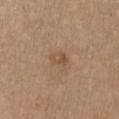Context:
The recorded lesion diameter is about 2.5 mm. The tile uses white-light illumination. This image is a 15 mm lesion crop taken from a total-body photograph. The patient is a female approximately 60 years of age. From the chest.